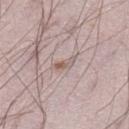Captured during whole-body skin photography for melanoma surveillance; the lesion was not biopsied.
A region of skin cropped from a whole-body photographic capture, roughly 15 mm wide.
The tile uses white-light illumination.
The patient is a male in their mid- to late 20s.
Located on the left lower leg.
Approximately 2.5 mm at its widest.